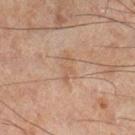Clinical impression:
This lesion was catalogued during total-body skin photography and was not selected for biopsy.
Context:
Automated image analysis of the tile measured an average lesion color of about L≈46 a*≈15 b*≈26 (CIELAB) and a normalized lesion–skin contrast near 4.5. It also reported a border-irregularity index near 5.5/10, internal color variation of about 1 on a 0–10 scale, and radial color variation of about 0.5. The analysis additionally found a nevus-likeness score of about 0/100. The tile uses cross-polarized illumination. A male patient in their mid-40s. A 15 mm close-up tile from a total-body photography series done for melanoma screening. Longest diameter approximately 3.5 mm. The lesion is located on the right lower leg.